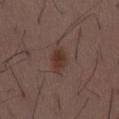The lesion was photographed on a routine skin check and not biopsied; there is no pathology result. A male subject aged 48 to 52. A 15 mm close-up extracted from a 3D total-body photography capture. Located on the lower back.